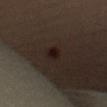The lesion was tiled from a total-body skin photograph and was not biopsied.